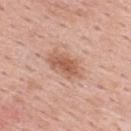The patient is a male aged 53–57.
Located on the upper back.
A 15 mm close-up extracted from a 3D total-body photography capture.
Captured under white-light illumination.
Longest diameter approximately 5 mm.